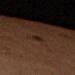Context:
A close-up tile cropped from a whole-body skin photograph, about 15 mm across. Located on the left thigh. The patient is a female aged 68 to 72.